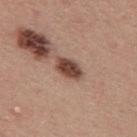follow-up: catalogued during a skin exam; not biopsied
lesion size: ≈3.5 mm
image source: ~15 mm tile from a whole-body skin photo
body site: the upper back
subject: male, in their 40s